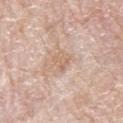notes: imaged on a skin check; not biopsied
illumination: white-light
lesion diameter: ~2.5 mm (longest diameter)
acquisition: ~15 mm crop, total-body skin-cancer survey
subject: male, about 80 years old
location: the right upper arm